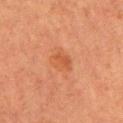The lesion was photographed on a routine skin check and not biopsied; there is no pathology result. Measured at roughly 3 mm in maximum diameter. Located on the arm. A roughly 15 mm field-of-view crop from a total-body skin photograph. A female patient, aged 53 to 57. Captured under cross-polarized illumination.